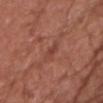Q: Was this lesion biopsied?
A: no biopsy performed (imaged during a skin exam)
Q: Where on the body is the lesion?
A: the chest
Q: Who is the patient?
A: male, in their mid- to late 70s
Q: What kind of image is this?
A: 15 mm crop, total-body photography